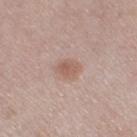workup = imaged on a skin check; not biopsied | tile lighting = white-light illumination | image source = ~15 mm tile from a whole-body skin photo | location = the right thigh | subject = female, aged 58–62 | TBP lesion metrics = a lesion area of about 5 mm², a shape eccentricity near 0.65, and a symmetry-axis asymmetry near 0.2; a border-irregularity index near 2/10 and peripheral color asymmetry of about 1; an automated nevus-likeness rating near 60 out of 100 and a lesion-detection confidence of about 100/100.